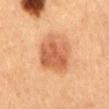{"biopsy_status": "not biopsied; imaged during a skin examination", "lesion_size": {"long_diameter_mm_approx": 5.0}, "automated_metrics": {"cielab_L": 48, "cielab_a": 22, "cielab_b": 32, "vs_skin_darker_L": 10.0, "color_variation_0_10": 3.5, "peripheral_color_asymmetry": 1.0}, "image": {"source": "total-body photography crop", "field_of_view_mm": 15}, "site": "chest", "patient": {"sex": "female", "age_approx": 60}, "lighting": "cross-polarized"}